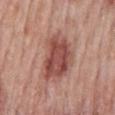biopsy status: no biopsy performed (imaged during a skin exam)
imaging modality: ~15 mm tile from a whole-body skin photo
site: the mid back
lighting: white-light
subject: male, aged around 50
automated metrics: an outline eccentricity of about 0.7 (0 = round, 1 = elongated) and a symmetry-axis asymmetry near 0.3; an average lesion color of about L≈49 a*≈25 b*≈25 (CIELAB), a lesion–skin lightness drop of about 13, and a lesion-to-skin contrast of about 9 (normalized; higher = more distinct); a border-irregularity index near 3/10
lesion size: ≈6 mm